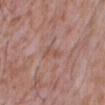Q: Is there a histopathology result?
A: total-body-photography surveillance lesion; no biopsy
Q: Where on the body is the lesion?
A: the chest
Q: Who is the patient?
A: male, about 45 years old
Q: How was this image acquired?
A: ~15 mm tile from a whole-body skin photo